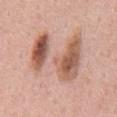The recorded lesion diameter is about 9.5 mm. Cropped from a whole-body photographic skin survey; the tile spans about 15 mm. On the back. The patient is a male approximately 55 years of age. Automated image analysis of the tile measured an average lesion color of about L≈61 a*≈21 b*≈28 (CIELAB) and about 10 CIELAB-L* units darker than the surrounding skin. The software also gave a border-irregularity rating of about 7/10, a color-variation rating of about 10/10, and radial color variation of about 5.